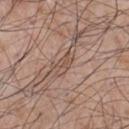{"biopsy_status": "not biopsied; imaged during a skin examination", "automated_metrics": {"nevus_likeness_0_100": 0, "lesion_detection_confidence_0_100": 50}, "site": "chest", "lighting": "white-light", "patient": {"sex": "male", "age_approx": 55}, "image": {"source": "total-body photography crop", "field_of_view_mm": 15}, "lesion_size": {"long_diameter_mm_approx": 3.0}}